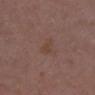Clinical impression: This lesion was catalogued during total-body skin photography and was not selected for biopsy. Image and clinical context: The tile uses white-light illumination. A 15 mm close-up tile from a total-body photography series done for melanoma screening. The subject is a female aged 28 to 32. Approximately 2.5 mm at its widest. From the chest.